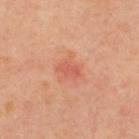Impression:
This lesion was catalogued during total-body skin photography and was not selected for biopsy.
Acquisition and patient details:
A female subject, aged around 40. Automated image analysis of the tile measured a lesion color around L≈58 a*≈32 b*≈33 in CIELAB, about 8 CIELAB-L* units darker than the surrounding skin, and a normalized lesion–skin contrast near 5. Cropped from a total-body skin-imaging series; the visible field is about 15 mm. This is a cross-polarized tile. Longest diameter approximately 2.5 mm. Located on the back.